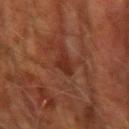Clinical impression:
No biopsy was performed on this lesion — it was imaged during a full skin examination and was not determined to be concerning.
Context:
The lesion is on the arm. The subject is a male approximately 70 years of age. The recorded lesion diameter is about 2.5 mm. A region of skin cropped from a whole-body photographic capture, roughly 15 mm wide. Automated image analysis of the tile measured a peripheral color-asymmetry measure near 0.5. The analysis additionally found a classifier nevus-likeness of about 5/100 and lesion-presence confidence of about 100/100.